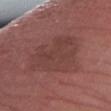The lesion was photographed on a routine skin check and not biopsied; there is no pathology result. On the arm. The total-body-photography lesion software estimated a mean CIELAB color near L≈41 a*≈22 b*≈22 and a lesion-to-skin contrast of about 5 (normalized; higher = more distinct). It also reported an automated nevus-likeness rating near 0 out of 100 and a lesion-detection confidence of about 100/100. About 6.5 mm across. A lesion tile, about 15 mm wide, cut from a 3D total-body photograph. A male patient about 75 years old.